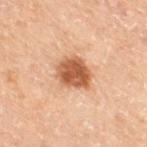Assessment: No biopsy was performed on this lesion — it was imaged during a full skin examination and was not determined to be concerning. Clinical summary: On the leg. A male patient aged around 70. A lesion tile, about 15 mm wide, cut from a 3D total-body photograph.